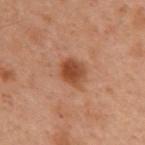Captured during whole-body skin photography for melanoma surveillance; the lesion was not biopsied.
This image is a 15 mm lesion crop taken from a total-body photograph.
The lesion is on the upper back.
Captured under cross-polarized illumination.
Longest diameter approximately 3 mm.
A female patient approximately 55 years of age.